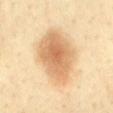- illumination — cross-polarized illumination
- site — the mid back
- diameter — ~7 mm (longest diameter)
- imaging modality — ~15 mm crop, total-body skin-cancer survey
- patient — female, aged around 40
- automated lesion analysis — a lesion area of about 26 mm²